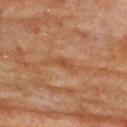• follow-up · imaged on a skin check; not biopsied
• acquisition · total-body-photography crop, ~15 mm field of view
• patient · female, aged 78–82
• body site · the upper back